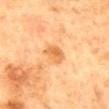No biopsy was performed on this lesion — it was imaged during a full skin examination and was not determined to be concerning. The recorded lesion diameter is about 2.5 mm. Cropped from a total-body skin-imaging series; the visible field is about 15 mm. A female patient, aged 58 to 62. On the mid back. The tile uses cross-polarized illumination.